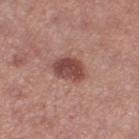biopsy status — imaged on a skin check; not biopsied
imaging modality — total-body-photography crop, ~15 mm field of view
illumination — white-light illumination
body site — the right thigh
subject — female, aged around 40